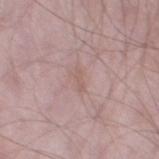biopsy status = catalogued during a skin exam; not biopsied | location = the left thigh | lesion size = ≈2.5 mm | tile lighting = white-light illumination | image source = ~15 mm tile from a whole-body skin photo | subject = male, aged approximately 55.